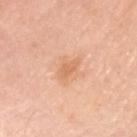<case>
  <biopsy_status>not biopsied; imaged during a skin examination</biopsy_status>
  <patient>
    <sex>female</sex>
    <age_approx>65</age_approx>
  </patient>
  <site>left upper arm</site>
  <image>
    <source>total-body photography crop</source>
    <field_of_view_mm>15</field_of_view_mm>
  </image>
  <lesion_size>
    <long_diameter_mm_approx>3.0</long_diameter_mm_approx>
  </lesion_size>
</case>